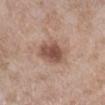Q: Is there a histopathology result?
A: no biopsy performed (imaged during a skin exam)
Q: What is the anatomic site?
A: the right lower leg
Q: Who is the patient?
A: female, roughly 55 years of age
Q: Lesion size?
A: ~4.5 mm (longest diameter)
Q: What lighting was used for the tile?
A: white-light
Q: What is the imaging modality?
A: ~15 mm crop, total-body skin-cancer survey